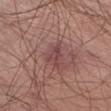notes = catalogued during a skin exam; not biopsied
patient = male, aged 78–82
image source = ~15 mm tile from a whole-body skin photo
body site = the abdomen